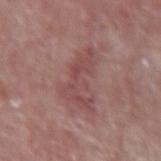Impression:
Part of a total-body skin-imaging series; this lesion was reviewed on a skin check and was not flagged for biopsy.
Clinical summary:
A female patient aged around 60. The total-body-photography lesion software estimated a footprint of about 9 mm² and a shape-asymmetry score of about 0.75 (0 = symmetric). The software also gave a border-irregularity rating of about 10/10, internal color variation of about 2 on a 0–10 scale, and radial color variation of about 0.5. And it measured a classifier nevus-likeness of about 0/100 and a detector confidence of about 100 out of 100 that the crop contains a lesion. Approximately 5.5 mm at its widest. From the right forearm. Cropped from a whole-body photographic skin survey; the tile spans about 15 mm.